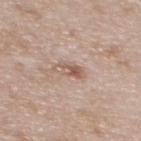<tbp_lesion>
  <biopsy_status>not biopsied; imaged during a skin examination</biopsy_status>
  <patient>
    <sex>female</sex>
    <age_approx>30</age_approx>
  </patient>
  <site>upper back</site>
  <image>
    <source>total-body photography crop</source>
    <field_of_view_mm>15</field_of_view_mm>
  </image>
</tbp_lesion>